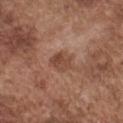<case>
<biopsy_status>not biopsied; imaged during a skin examination</biopsy_status>
<site>chest</site>
<patient>
  <sex>male</sex>
  <age_approx>75</age_approx>
</patient>
<lighting>white-light</lighting>
<lesion_size>
  <long_diameter_mm_approx>3.0</long_diameter_mm_approx>
</lesion_size>
<automated_metrics>
  <cielab_L>46</cielab_L>
  <cielab_a>21</cielab_a>
  <cielab_b>28</cielab_b>
  <vs_skin_contrast_norm>7.0</vs_skin_contrast_norm>
  <color_variation_0_10>3.5</color_variation_0_10>
  <peripheral_color_asymmetry>1.5</peripheral_color_asymmetry>
</automated_metrics>
<image>
  <source>total-body photography crop</source>
  <field_of_view_mm>15</field_of_view_mm>
</image>
</case>